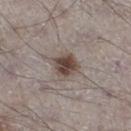– follow-up · catalogued during a skin exam; not biopsied
– patient · male, aged 68 to 72
– imaging modality · 15 mm crop, total-body photography
– anatomic site · the left lower leg
– size · ~3 mm (longest diameter)